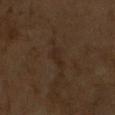Impression: Imaged during a routine full-body skin examination; the lesion was not biopsied and no histopathology is available. Acquisition and patient details: A male patient in their mid- to late 60s. A 15 mm close-up extracted from a 3D total-body photography capture. Captured under cross-polarized illumination. An algorithmic analysis of the crop reported an average lesion color of about L≈23 a*≈15 b*≈22 (CIELAB), roughly 5 lightness units darker than nearby skin, and a lesion-to-skin contrast of about 6 (normalized; higher = more distinct). The analysis additionally found a classifier nevus-likeness of about 0/100 and lesion-presence confidence of about 75/100. About 2.5 mm across.